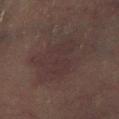| feature | finding |
|---|---|
| lesion diameter | about 5.5 mm |
| subject | male, aged 58 to 62 |
| illumination | cross-polarized illumination |
| acquisition | 15 mm crop, total-body photography |